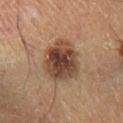Q: Was a biopsy performed?
A: no biopsy performed (imaged during a skin exam)
Q: What kind of image is this?
A: ~15 mm crop, total-body skin-cancer survey
Q: What are the patient's age and sex?
A: male, aged 58 to 62
Q: Lesion location?
A: the right lower leg
Q: Lesion size?
A: about 5.5 mm
Q: What lighting was used for the tile?
A: cross-polarized illumination